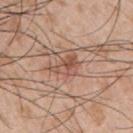Clinical impression:
This lesion was catalogued during total-body skin photography and was not selected for biopsy.
Background:
A lesion tile, about 15 mm wide, cut from a 3D total-body photograph. The patient is a male aged approximately 55. Located on the right upper arm.